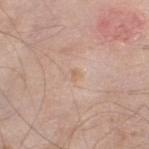follow-up = imaged on a skin check; not biopsied
lighting = white-light illumination
patient = male, aged 58 to 62
diameter = ≈1.5 mm
image-analysis metrics = a lesion color around L≈63 a*≈17 b*≈31 in CIELAB, a lesion–skin lightness drop of about 6, and a normalized lesion–skin contrast near 5.5; a border-irregularity rating of about 3.5/10 and a color-variation rating of about 0/10; a nevus-likeness score of about 0/100 and lesion-presence confidence of about 100/100
body site = the leg
image source = total-body-photography crop, ~15 mm field of view